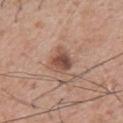Notes:
• follow-up: catalogued during a skin exam; not biopsied
• size: ~3.5 mm (longest diameter)
• location: the upper back
• imaging modality: ~15 mm crop, total-body skin-cancer survey
• patient: male, aged 63 to 67
• image-analysis metrics: a footprint of about 6 mm², an eccentricity of roughly 0.6, and a shape-asymmetry score of about 0.2 (0 = symmetric); a color-variation rating of about 5.5/10 and a peripheral color-asymmetry measure near 2